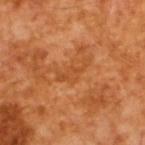Impression:
The lesion was tiled from a total-body skin photograph and was not biopsied.
Image and clinical context:
A male patient aged 63 to 67. This is a cross-polarized tile. Cropped from a whole-body photographic skin survey; the tile spans about 15 mm.